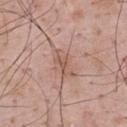<tbp_lesion>
  <biopsy_status>not biopsied; imaged during a skin examination</biopsy_status>
  <automated_metrics>
    <area_mm2_approx>5.5</area_mm2_approx>
    <eccentricity>0.85</eccentricity>
    <shape_asymmetry>0.35</shape_asymmetry>
    <cielab_L>56</cielab_L>
    <cielab_a>20</cielab_a>
    <cielab_b>26</cielab_b>
    <vs_skin_darker_L>8.0</vs_skin_darker_L>
    <vs_skin_contrast_norm>5.5</vs_skin_contrast_norm>
    <border_irregularity_0_10>4.0</border_irregularity_0_10>
    <peripheral_color_asymmetry>1.0</peripheral_color_asymmetry>
  </automated_metrics>
  <patient>
    <sex>male</sex>
    <age_approx>55</age_approx>
  </patient>
  <lesion_size>
    <long_diameter_mm_approx>3.5</long_diameter_mm_approx>
  </lesion_size>
  <site>upper back</site>
  <image>
    <source>total-body photography crop</source>
    <field_of_view_mm>15</field_of_view_mm>
  </image>
  <lighting>white-light</lighting>
</tbp_lesion>